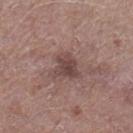The lesion was photographed on a routine skin check and not biopsied; there is no pathology result. A male patient, approximately 80 years of age. Approximately 3.5 mm at its widest. On the left leg. An algorithmic analysis of the crop reported a lesion color around L≈44 a*≈19 b*≈19 in CIELAB, roughly 9 lightness units darker than nearby skin, and a lesion-to-skin contrast of about 7.5 (normalized; higher = more distinct). The software also gave internal color variation of about 3.5 on a 0–10 scale and radial color variation of about 1. And it measured a lesion-detection confidence of about 100/100. A 15 mm crop from a total-body photograph taken for skin-cancer surveillance.